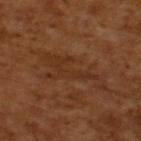The lesion was photographed on a routine skin check and not biopsied; there is no pathology result. The lesion's longest dimension is about 7.5 mm. A 15 mm close-up extracted from a 3D total-body photography capture. A male patient aged 63–67. The tile uses cross-polarized illumination.